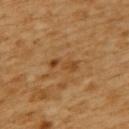A roughly 15 mm field-of-view crop from a total-body skin photograph. A female subject, aged around 55. Automated tile analysis of the lesion measured a footprint of about 4 mm², an outline eccentricity of about 0.9 (0 = round, 1 = elongated), and two-axis asymmetry of about 0.3. It also reported a color-variation rating of about 1/10 and a peripheral color-asymmetry measure near 0. Imaged with cross-polarized lighting. About 3.5 mm across. On the upper back.